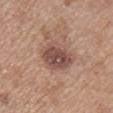follow-up = imaged on a skin check; not biopsied
image-analysis metrics = a border-irregularity index near 2/10, internal color variation of about 5 on a 0–10 scale, and a peripheral color-asymmetry measure near 2; an automated nevus-likeness rating near 35 out of 100 and a detector confidence of about 100 out of 100 that the crop contains a lesion
patient = female, approximately 40 years of age
imaging modality = 15 mm crop, total-body photography
body site = the right upper arm